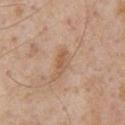Part of a total-body skin-imaging series; this lesion was reviewed on a skin check and was not flagged for biopsy. A lesion tile, about 15 mm wide, cut from a 3D total-body photograph. The lesion is located on the chest. The subject is a male approximately 60 years of age.